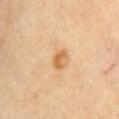Imaged with cross-polarized lighting.
Cropped from a whole-body photographic skin survey; the tile spans about 15 mm.
A female subject, aged around 60.
Located on the chest.
Approximately 2.5 mm at its widest.
The lesion-visualizer software estimated a lesion area of about 4 mm², a shape eccentricity near 0.75, and two-axis asymmetry of about 0.35. And it measured peripheral color asymmetry of about 1.